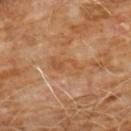  biopsy_status: not biopsied; imaged during a skin examination
  lesion_size:
    long_diameter_mm_approx: 4.0
  patient:
    sex: male
    age_approx: 60
  site: chest
  automated_metrics:
    cielab_L: 50
    cielab_a: 21
    cielab_b: 36
    vs_skin_darker_L: 6.0
    vs_skin_contrast_norm: 5.5
    color_variation_0_10: 3.5
    peripheral_color_asymmetry: 1.5
    nevus_likeness_0_100: 0
    lesion_detection_confidence_0_100: 100
  lighting: cross-polarized
  image:
    source: total-body photography crop
    field_of_view_mm: 15